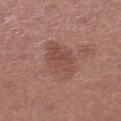Q: Was a biopsy performed?
A: imaged on a skin check; not biopsied
Q: Where on the body is the lesion?
A: the left lower leg
Q: How was the tile lit?
A: white-light illumination
Q: Who is the patient?
A: female, aged 48 to 52
Q: What did automated image analysis measure?
A: an eccentricity of roughly 0.6; border irregularity of about 3 on a 0–10 scale, internal color variation of about 3.5 on a 0–10 scale, and radial color variation of about 1.5
Q: What kind of image is this?
A: total-body-photography crop, ~15 mm field of view
Q: How large is the lesion?
A: about 4.5 mm